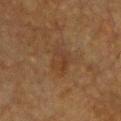notes=catalogued during a skin exam; not biopsied | imaging modality=~15 mm tile from a whole-body skin photo | site=the chest | subject=female, roughly 55 years of age.